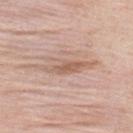Clinical impression: No biopsy was performed on this lesion — it was imaged during a full skin examination and was not determined to be concerning. Context: Cropped from a total-body skin-imaging series; the visible field is about 15 mm. A male subject, in their mid-30s. Approximately 6.5 mm at its widest. On the back. Captured under white-light illumination.